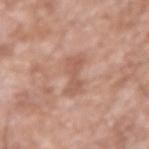biopsy status: no biopsy performed (imaged during a skin exam)
TBP lesion metrics: a lesion area of about 5.5 mm² and a symmetry-axis asymmetry near 0.35; a classifier nevus-likeness of about 0/100
lighting: white-light
location: the arm
diameter: ≈4.5 mm
acquisition: ~15 mm tile from a whole-body skin photo
subject: male, in their 60s